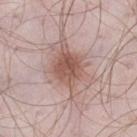workup — catalogued during a skin exam; not biopsied
body site — the left thigh
lighting — white-light
imaging modality — ~15 mm tile from a whole-body skin photo
subject — male, aged 53–57
diameter — ≈7.5 mm